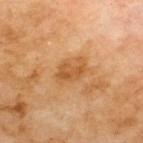Assessment:
This lesion was catalogued during total-body skin photography and was not selected for biopsy.
Context:
The total-body-photography lesion software estimated an outline eccentricity of about 0.8 (0 = round, 1 = elongated). And it measured a lesion color around L≈54 a*≈24 b*≈42 in CIELAB, a lesion–skin lightness drop of about 9, and a lesion-to-skin contrast of about 7 (normalized; higher = more distinct). The patient is a male aged around 70. A roughly 15 mm field-of-view crop from a total-body skin photograph. The lesion is on the upper back.